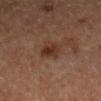Captured under cross-polarized illumination.
A female patient aged 43 to 47.
An algorithmic analysis of the crop reported a shape eccentricity near 0.6 and a shape-asymmetry score of about 0.25 (0 = symmetric).
The lesion is located on the right upper arm.
Measured at roughly 3 mm in maximum diameter.
A 15 mm close-up tile from a total-body photography series done for melanoma screening.